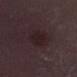| key | value |
|---|---|
| biopsy status | imaged on a skin check; not biopsied |
| image source | ~15 mm crop, total-body skin-cancer survey |
| patient | male, aged around 50 |
| lesion size | ≈4 mm |